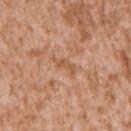{"biopsy_status": "not biopsied; imaged during a skin examination", "site": "left upper arm", "patient": {"sex": "male", "age_approx": 45}, "image": {"source": "total-body photography crop", "field_of_view_mm": 15}}